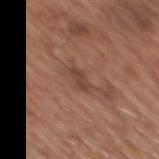- diameter · about 3 mm
- location · the mid back
- image · 15 mm crop, total-body photography
- subject · male, about 65 years old